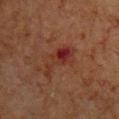{
  "biopsy_status": "not biopsied; imaged during a skin examination",
  "site": "chest",
  "lesion_size": {
    "long_diameter_mm_approx": 5.5
  },
  "image": {
    "source": "total-body photography crop",
    "field_of_view_mm": 15
  },
  "patient": {
    "sex": "male",
    "age_approx": 65
  },
  "lighting": "cross-polarized"
}